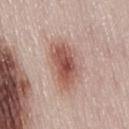Automated tile analysis of the lesion measured a lesion color around L≈54 a*≈23 b*≈26 in CIELAB, about 13 CIELAB-L* units darker than the surrounding skin, and a normalized border contrast of about 9.
A female patient about 50 years old.
Measured at roughly 5.5 mm in maximum diameter.
Located on the lower back.
This image is a 15 mm lesion crop taken from a total-body photograph.
The tile uses white-light illumination.